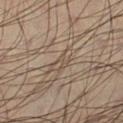{"biopsy_status": "not biopsied; imaged during a skin examination", "lighting": "cross-polarized", "site": "left lower leg", "image": {"source": "total-body photography crop", "field_of_view_mm": 15}, "patient": {"sex": "male", "age_approx": 45}, "automated_metrics": {"cielab_L": 49, "cielab_a": 12, "cielab_b": 24, "vs_skin_darker_L": 6.0, "border_irregularity_0_10": 4.0, "color_variation_0_10": 2.0, "nevus_likeness_0_100": 0}}